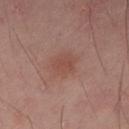<record>
  <biopsy_status>not biopsied; imaged during a skin examination</biopsy_status>
  <site>lower back</site>
  <patient>
    <sex>male</sex>
    <age_approx>40</age_approx>
  </patient>
  <lesion_size>
    <long_diameter_mm_approx>3.5</long_diameter_mm_approx>
  </lesion_size>
  <automated_metrics>
    <area_mm2_approx>7.5</area_mm2_approx>
    <eccentricity>0.5</eccentricity>
    <shape_asymmetry>0.2</shape_asymmetry>
    <border_irregularity_0_10>2.0</border_irregularity_0_10>
    <peripheral_color_asymmetry>0.5</peripheral_color_asymmetry>
    <nevus_likeness_0_100>15</nevus_likeness_0_100>
    <lesion_detection_confidence_0_100>100</lesion_detection_confidence_0_100>
  </automated_metrics>
  <image>
    <source>total-body photography crop</source>
    <field_of_view_mm>15</field_of_view_mm>
  </image>
  <lighting>cross-polarized</lighting>
</record>